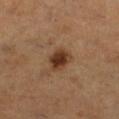Captured during whole-body skin photography for melanoma surveillance; the lesion was not biopsied. This is a cross-polarized tile. Automated tile analysis of the lesion measured a footprint of about 6.5 mm², an outline eccentricity of about 0.5 (0 = round, 1 = elongated), and two-axis asymmetry of about 0.15. It also reported a detector confidence of about 100 out of 100 that the crop contains a lesion. A 15 mm close-up tile from a total-body photography series done for melanoma screening. From the leg. A female subject, in their mid-50s.